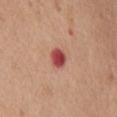follow-up: total-body-photography surveillance lesion; no biopsy | acquisition: ~15 mm tile from a whole-body skin photo | tile lighting: white-light illumination | automated metrics: an average lesion color of about L≈49 a*≈34 b*≈27 (CIELAB), about 16 CIELAB-L* units darker than the surrounding skin, and a lesion-to-skin contrast of about 11 (normalized; higher = more distinct); an automated nevus-likeness rating near 0 out of 100 and lesion-presence confidence of about 100/100 | patient: female, about 75 years old | lesion size: ≈2.5 mm | anatomic site: the chest.